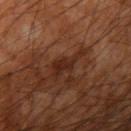The lesion was tiled from a total-body skin photograph and was not biopsied. The lesion is on the arm. A lesion tile, about 15 mm wide, cut from a 3D total-body photograph. A male patient, roughly 60 years of age.